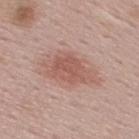follow-up = total-body-photography surveillance lesion; no biopsy
image source = total-body-photography crop, ~15 mm field of view
anatomic site = the upper back
patient = male, in their 60s